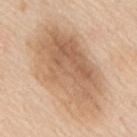Impression:
This lesion was catalogued during total-body skin photography and was not selected for biopsy.
Image and clinical context:
A lesion tile, about 15 mm wide, cut from a 3D total-body photograph. The total-body-photography lesion software estimated a lesion area of about 50 mm², an eccentricity of roughly 0.85, and a shape-asymmetry score of about 0.25 (0 = symmetric). Measured at roughly 11.5 mm in maximum diameter. A male subject, in their mid-60s. This is a white-light tile. The lesion is located on the upper back.